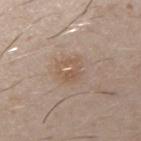No biopsy was performed on this lesion — it was imaged during a full skin examination and was not determined to be concerning.
Located on the right upper arm.
A region of skin cropped from a whole-body photographic capture, roughly 15 mm wide.
The lesion-visualizer software estimated an eccentricity of roughly 0.45 and two-axis asymmetry of about 0.2. The analysis additionally found a mean CIELAB color near L≈56 a*≈16 b*≈28, roughly 6 lightness units darker than nearby skin, and a lesion-to-skin contrast of about 5 (normalized; higher = more distinct). And it measured a nevus-likeness score of about 0/100 and a detector confidence of about 100 out of 100 that the crop contains a lesion.
A male patient, aged 28 to 32.
Captured under white-light illumination.